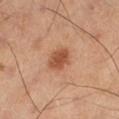Q: Is there a histopathology result?
A: no biopsy performed (imaged during a skin exam)
Q: Patient demographics?
A: male, aged approximately 60
Q: What did automated image analysis measure?
A: a footprint of about 6.5 mm², a shape eccentricity near 0.6, and two-axis asymmetry of about 0.2; a classifier nevus-likeness of about 90/100 and lesion-presence confidence of about 100/100
Q: Lesion size?
A: ~3 mm (longest diameter)
Q: What kind of image is this?
A: ~15 mm tile from a whole-body skin photo
Q: Lesion location?
A: the leg